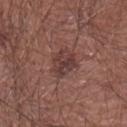The lesion was photographed on a routine skin check and not biopsied; there is no pathology result. A male subject, aged 43 to 47. The recorded lesion diameter is about 3.5 mm. Located on the right forearm. A 15 mm close-up extracted from a 3D total-body photography capture.